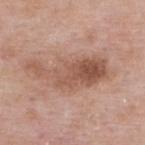follow-up = catalogued during a skin exam; not biopsied | image-analysis metrics = a mean CIELAB color near L≈54 a*≈21 b*≈28 and a normalized lesion–skin contrast near 7.5; border irregularity of about 5.5 on a 0–10 scale, a within-lesion color-variation index near 5.5/10, and peripheral color asymmetry of about 2 | image = ~15 mm tile from a whole-body skin photo | tile lighting = white-light illumination | patient = male, about 55 years old | site = the upper back | lesion size = about 9 mm.